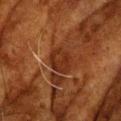| key | value |
|---|---|
| biopsy status | catalogued during a skin exam; not biopsied |
| anatomic site | the head or neck |
| image-analysis metrics | border irregularity of about 1.5 on a 0–10 scale, a within-lesion color-variation index near 3/10, and a peripheral color-asymmetry measure near 1; a nevus-likeness score of about 0/100 and a lesion-detection confidence of about 55/100 |
| patient | male, aged 78–82 |
| image source | ~15 mm tile from a whole-body skin photo |
| illumination | cross-polarized |
| lesion size | about 3.5 mm |